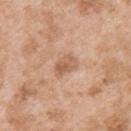notes = total-body-photography surveillance lesion; no biopsy | site = the back | lesion diameter = ~3 mm (longest diameter) | image = ~15 mm crop, total-body skin-cancer survey | image-analysis metrics = an average lesion color of about L≈60 a*≈21 b*≈33 (CIELAB), about 9 CIELAB-L* units darker than the surrounding skin, and a normalized lesion–skin contrast near 6; a border-irregularity index near 2.5/10 and radial color variation of about 1.5; a detector confidence of about 100 out of 100 that the crop contains a lesion | illumination = white-light | subject = male, aged 23 to 27.